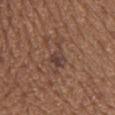{
  "biopsy_status": "not biopsied; imaged during a skin examination",
  "lighting": "white-light",
  "patient": {
    "sex": "male",
    "age_approx": 65
  },
  "site": "upper back",
  "image": {
    "source": "total-body photography crop",
    "field_of_view_mm": 15
  },
  "automated_metrics": {
    "eccentricity": 0.9,
    "shape_asymmetry": 0.5,
    "cielab_L": 41,
    "cielab_a": 18,
    "cielab_b": 23,
    "vs_skin_darker_L": 7.0,
    "vs_skin_contrast_norm": 7.0,
    "border_irregularity_0_10": 6.5,
    "color_variation_0_10": 5.0,
    "peripheral_color_asymmetry": 1.5
  },
  "lesion_size": {
    "long_diameter_mm_approx": 5.5
  }
}